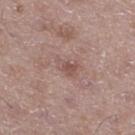Findings:
– biopsy status — catalogued during a skin exam; not biopsied
– body site — the leg
– patient — male, aged 48–52
– lighting — white-light
– image-analysis metrics — a footprint of about 3.5 mm², a shape eccentricity near 0.8, and two-axis asymmetry of about 0.4; an average lesion color of about L≈51 a*≈19 b*≈23 (CIELAB), about 8 CIELAB-L* units darker than the surrounding skin, and a normalized border contrast of about 6; border irregularity of about 3.5 on a 0–10 scale and a within-lesion color-variation index near 1.5/10
– lesion size — ~2.5 mm (longest diameter)
– imaging modality — 15 mm crop, total-body photography